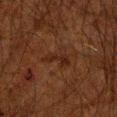  image:
    source: total-body photography crop
    field_of_view_mm: 15
  lighting: cross-polarized
  lesion_size:
    long_diameter_mm_approx: 4.0
  patient:
    sex: male
    age_approx: 60
  site: right upper arm